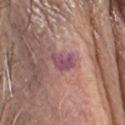{"biopsy_status": "not biopsied; imaged during a skin examination", "site": "head or neck", "patient": {"sex": "male", "age_approx": 80}, "image": {"source": "total-body photography crop", "field_of_view_mm": 15}}